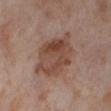Image and clinical context:
The patient is a female in their mid-50s. Captured under cross-polarized illumination. The lesion is on the right lower leg. A 15 mm crop from a total-body photograph taken for skin-cancer surveillance. Longest diameter approximately 7.5 mm.